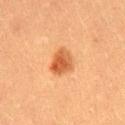No biopsy was performed on this lesion — it was imaged during a full skin examination and was not determined to be concerning.
Located on the lower back.
About 3.5 mm across.
A 15 mm close-up extracted from a 3D total-body photography capture.
A male subject aged 48–52.